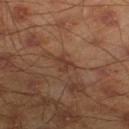<case>
  <biopsy_status>not biopsied; imaged during a skin examination</biopsy_status>
  <image>
    <source>total-body photography crop</source>
    <field_of_view_mm>15</field_of_view_mm>
  </image>
  <lighting>cross-polarized</lighting>
  <site>right lower leg</site>
  <patient>
    <sex>male</sex>
    <age_approx>45</age_approx>
  </patient>
</case>